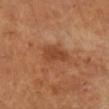Q: Was this lesion biopsied?
A: total-body-photography surveillance lesion; no biopsy
Q: How was this image acquired?
A: ~15 mm tile from a whole-body skin photo
Q: What did automated image analysis measure?
A: an average lesion color of about L≈45 a*≈25 b*≈35 (CIELAB) and a normalized lesion–skin contrast near 7; an automated nevus-likeness rating near 0 out of 100
Q: How large is the lesion?
A: ≈4 mm
Q: How was the tile lit?
A: cross-polarized
Q: What are the patient's age and sex?
A: female, aged 53 to 57
Q: Lesion location?
A: the right lower leg